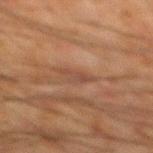biopsy status — no biopsy performed (imaged during a skin exam).